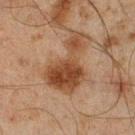From the right lower leg. A male subject aged around 45. Cropped from a whole-body photographic skin survey; the tile spans about 15 mm. This is a cross-polarized tile. The lesion's longest dimension is about 7 mm. Automated tile analysis of the lesion measured a lesion area of about 18 mm², an eccentricity of roughly 0.85, and a symmetry-axis asymmetry near 0.65. The software also gave an average lesion color of about L≈36 a*≈18 b*≈28 (CIELAB) and roughly 11 lightness units darker than nearby skin. The analysis additionally found a classifier nevus-likeness of about 55/100.